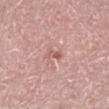Clinical impression:
No biopsy was performed on this lesion — it was imaged during a full skin examination and was not determined to be concerning.
Acquisition and patient details:
Longest diameter approximately 2.5 mm. The subject is a male aged 48 to 52. From the right lower leg. Imaged with white-light lighting. Cropped from a total-body skin-imaging series; the visible field is about 15 mm.